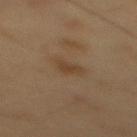Q: Was a biopsy performed?
A: total-body-photography surveillance lesion; no biopsy
Q: What is the anatomic site?
A: the mid back
Q: How large is the lesion?
A: ~3 mm (longest diameter)
Q: Automated lesion metrics?
A: a footprint of about 4 mm², an eccentricity of roughly 0.75, and two-axis asymmetry of about 0.35; a border-irregularity rating of about 3/10, a color-variation rating of about 1/10, and peripheral color asymmetry of about 0.5; a nevus-likeness score of about 5/100
Q: What kind of image is this?
A: total-body-photography crop, ~15 mm field of view
Q: What are the patient's age and sex?
A: male, approximately 55 years of age